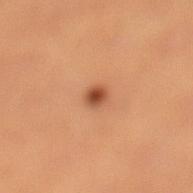{"biopsy_status": "not biopsied; imaged during a skin examination", "lighting": "cross-polarized", "site": "left lower leg", "image": {"source": "total-body photography crop", "field_of_view_mm": 15}, "patient": {"sex": "female", "age_approx": 35}, "lesion_size": {"long_diameter_mm_approx": 2.0}}